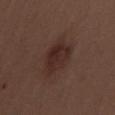Image and clinical context:
An algorithmic analysis of the crop reported border irregularity of about 2.5 on a 0–10 scale, a color-variation rating of about 3/10, and a peripheral color-asymmetry measure near 1. This image is a 15 mm lesion crop taken from a total-body photograph. On the right thigh. Approximately 5.5 mm at its widest. A female patient aged around 40.